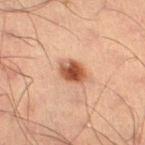notes: no biopsy performed (imaged during a skin exam)
patient: male, aged approximately 55
image: 15 mm crop, total-body photography
body site: the leg
illumination: cross-polarized